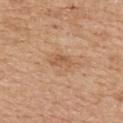Impression: The lesion was photographed on a routine skin check and not biopsied; there is no pathology result. Background: Imaged with white-light lighting. The lesion is located on the back. This image is a 15 mm lesion crop taken from a total-body photograph. A female patient in their mid-50s. Longest diameter approximately 3 mm.